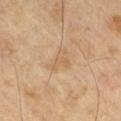Q: Was this lesion biopsied?
A: no biopsy performed (imaged during a skin exam)
Q: What lighting was used for the tile?
A: cross-polarized
Q: What is the lesion's diameter?
A: ~3.5 mm (longest diameter)
Q: How was this image acquired?
A: total-body-photography crop, ~15 mm field of view
Q: Lesion location?
A: the leg
Q: Patient demographics?
A: male, aged approximately 70